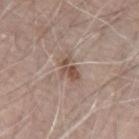Notes:
– body site · the left upper arm
– patient · male, approximately 70 years of age
– image source · ~15 mm crop, total-body skin-cancer survey